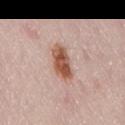follow-up = catalogued during a skin exam; not biopsied
image source = ~15 mm tile from a whole-body skin photo
anatomic site = the lower back
patient = female, aged around 50
lesion diameter = ≈4.5 mm
lighting = white-light